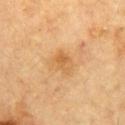<tbp_lesion>
  <biopsy_status>not biopsied; imaged during a skin examination</biopsy_status>
  <site>front of the torso</site>
  <image>
    <source>total-body photography crop</source>
    <field_of_view_mm>15</field_of_view_mm>
  </image>
  <patient>
    <sex>male</sex>
    <age_approx>85+</age_approx>
  </patient>
  <lighting>cross-polarized</lighting>
  <lesion_size>
    <long_diameter_mm_approx>2.5</long_diameter_mm_approx>
  </lesion_size>
</tbp_lesion>